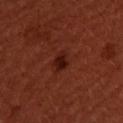<case>
<biopsy_status>not biopsied; imaged during a skin examination</biopsy_status>
<image>
  <source>total-body photography crop</source>
  <field_of_view_mm>15</field_of_view_mm>
</image>
<lighting>cross-polarized</lighting>
<patient>
  <sex>female</sex>
  <age_approx>50</age_approx>
</patient>
</case>